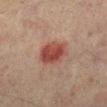Clinical impression: This lesion was catalogued during total-body skin photography and was not selected for biopsy. Clinical summary: The recorded lesion diameter is about 4 mm. The tile uses cross-polarized illumination. A male patient roughly 75 years of age. From the right lower leg. Cropped from a whole-body photographic skin survey; the tile spans about 15 mm. The total-body-photography lesion software estimated a lesion color around L≈39 a*≈23 b*≈23 in CIELAB, roughly 10 lightness units darker than nearby skin, and a lesion-to-skin contrast of about 9 (normalized; higher = more distinct). And it measured border irregularity of about 2 on a 0–10 scale, a within-lesion color-variation index near 4.5/10, and radial color variation of about 1.5.